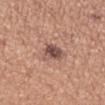Q: Was a biopsy performed?
A: no biopsy performed (imaged during a skin exam)
Q: How was the tile lit?
A: white-light
Q: Lesion size?
A: about 3.5 mm
Q: What is the anatomic site?
A: the left forearm
Q: Who is the patient?
A: female, aged 53 to 57
Q: What is the imaging modality?
A: ~15 mm crop, total-body skin-cancer survey